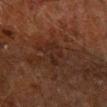Context:
Imaged with cross-polarized lighting. A male patient, about 60 years old. The lesion is on the right forearm. A region of skin cropped from a whole-body photographic capture, roughly 15 mm wide.